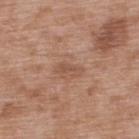{
  "biopsy_status": "not biopsied; imaged during a skin examination",
  "lesion_size": {
    "long_diameter_mm_approx": 2.5
  },
  "automated_metrics": {
    "area_mm2_approx": 2.5,
    "cielab_L": 52,
    "cielab_a": 21,
    "cielab_b": 29,
    "vs_skin_contrast_norm": 5.5,
    "nevus_likeness_0_100": 0,
    "lesion_detection_confidence_0_100": 100
  },
  "site": "upper back",
  "image": {
    "source": "total-body photography crop",
    "field_of_view_mm": 15
  },
  "patient": {
    "sex": "male",
    "age_approx": 50
  },
  "lighting": "white-light"
}